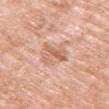<case>
  <biopsy_status>not biopsied; imaged during a skin examination</biopsy_status>
  <image>
    <source>total-body photography crop</source>
    <field_of_view_mm>15</field_of_view_mm>
  </image>
  <site>chest</site>
  <patient>
    <sex>female</sex>
    <age_approx>70</age_approx>
  </patient>
</case>